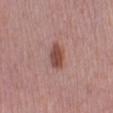No biopsy was performed on this lesion — it was imaged during a full skin examination and was not determined to be concerning. A 15 mm close-up extracted from a 3D total-body photography capture. Longest diameter approximately 3.5 mm. Imaged with white-light lighting. Located on the left lower leg. The subject is a female in their 40s.